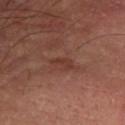biopsy status: total-body-photography surveillance lesion; no biopsy | image: 15 mm crop, total-body photography | subject: male, approximately 65 years of age | anatomic site: the left forearm.